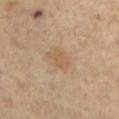Imaged during a routine full-body skin examination; the lesion was not biopsied and no histopathology is available.
The lesion is located on the back.
Cropped from a total-body skin-imaging series; the visible field is about 15 mm.
A female patient approximately 75 years of age.
Captured under cross-polarized illumination.
Measured at roughly 3 mm in maximum diameter.